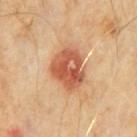A male subject, aged around 60.
Automated image analysis of the tile measured a lesion area of about 14 mm², an outline eccentricity of about 0.7 (0 = round, 1 = elongated), and a symmetry-axis asymmetry near 0.25.
Located on the abdomen.
This is a cross-polarized tile.
This image is a 15 mm lesion crop taken from a total-body photograph.
Histopathology of the biopsied lesion showed a nodular basal cell carcinoma (malignant).